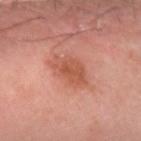The lesion was tiled from a total-body skin photograph and was not biopsied. Cropped from a total-body skin-imaging series; the visible field is about 15 mm. On the arm. A male subject, aged approximately 60.